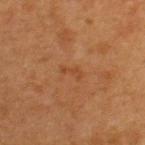An algorithmic analysis of the crop reported an automated nevus-likeness rating near 0 out of 100 and a detector confidence of about 100 out of 100 that the crop contains a lesion.
The patient is a female aged around 40.
The lesion is located on the upper back.
This is a cross-polarized tile.
Cropped from a whole-body photographic skin survey; the tile spans about 15 mm.
The recorded lesion diameter is about 3 mm.